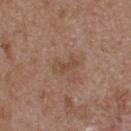Part of a total-body skin-imaging series; this lesion was reviewed on a skin check and was not flagged for biopsy. The recorded lesion diameter is about 3.5 mm. This is a white-light tile. The lesion is on the upper back. The patient is a male aged around 50. An algorithmic analysis of the crop reported an area of roughly 3.5 mm², a shape eccentricity near 0.9, and a shape-asymmetry score of about 0.45 (0 = symmetric). And it measured about 7 CIELAB-L* units darker than the surrounding skin and a normalized border contrast of about 5.5. The analysis additionally found border irregularity of about 5 on a 0–10 scale and internal color variation of about 1 on a 0–10 scale. Cropped from a total-body skin-imaging series; the visible field is about 15 mm.